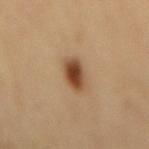Context:
The tile uses cross-polarized illumination. A female patient aged approximately 70. The total-body-photography lesion software estimated an average lesion color of about L≈47 a*≈20 b*≈35 (CIELAB) and roughly 16 lightness units darker than nearby skin. And it measured a detector confidence of about 100 out of 100 that the crop contains a lesion. Longest diameter approximately 4 mm. From the mid back. A 15 mm crop from a total-body photograph taken for skin-cancer surveillance.